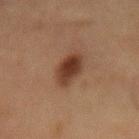{
  "biopsy_status": "not biopsied; imaged during a skin examination",
  "patient": {
    "sex": "male",
    "age_approx": 65
  },
  "image": {
    "source": "total-body photography crop",
    "field_of_view_mm": 15
  },
  "lesion_size": {
    "long_diameter_mm_approx": 4.0
  },
  "site": "lower back",
  "automated_metrics": {
    "cielab_L": 30,
    "cielab_a": 18,
    "cielab_b": 24,
    "vs_skin_darker_L": 11.0,
    "vs_skin_contrast_norm": 11.0,
    "nevus_likeness_0_100": 100,
    "lesion_detection_confidence_0_100": 100
  }
}